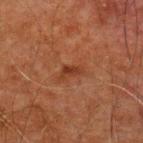  biopsy_status: not biopsied; imaged during a skin examination
  lesion_size:
    long_diameter_mm_approx: 2.5
  patient:
    sex: male
    age_approx: 60
  site: upper back
  automated_metrics:
    area_mm2_approx: 2.5
    eccentricity: 0.85
    color_variation_0_10: 0.0
    peripheral_color_asymmetry: 0.0
  image:
    source: total-body photography crop
    field_of_view_mm: 15